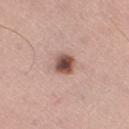<case>
<biopsy_status>not biopsied; imaged during a skin examination</biopsy_status>
<lighting>white-light</lighting>
<patient>
  <sex>male</sex>
  <age_approx>60</age_approx>
</patient>
<site>right thigh</site>
<image>
  <source>total-body photography crop</source>
  <field_of_view_mm>15</field_of_view_mm>
</image>
<lesion_size>
  <long_diameter_mm_approx>3.0</long_diameter_mm_approx>
</lesion_size>
</case>